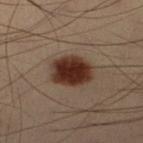  image:
    source: total-body photography crop
    field_of_view_mm: 15
  lesion_size:
    long_diameter_mm_approx: 4.5
  site: right lower leg
  patient:
    sex: male
    age_approx: 55
  lighting: cross-polarized